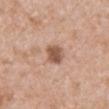This lesion was catalogued during total-body skin photography and was not selected for biopsy. This image is a 15 mm lesion crop taken from a total-body photograph. An algorithmic analysis of the crop reported an area of roughly 5.5 mm², an eccentricity of roughly 0.6, and a symmetry-axis asymmetry near 0.2. It also reported internal color variation of about 3.5 on a 0–10 scale and radial color variation of about 1. The software also gave a classifier nevus-likeness of about 80/100. The recorded lesion diameter is about 2.5 mm. The lesion is located on the mid back. The tile uses white-light illumination. A male subject in their 60s.